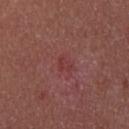body site=the upper back
acquisition=~15 mm crop, total-body skin-cancer survey
subject=female, about 25 years old